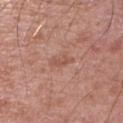Q: Is there a histopathology result?
A: imaged on a skin check; not biopsied
Q: Automated lesion metrics?
A: a footprint of about 2.5 mm², an eccentricity of roughly 0.9, and two-axis asymmetry of about 0.35; a border-irregularity rating of about 4/10 and internal color variation of about 0 on a 0–10 scale
Q: How large is the lesion?
A: ≈2.5 mm
Q: Patient demographics?
A: male, aged 48–52
Q: How was the tile lit?
A: white-light illumination
Q: What is the imaging modality?
A: ~15 mm tile from a whole-body skin photo
Q: What is the anatomic site?
A: the left upper arm